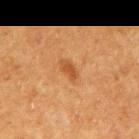This lesion was catalogued during total-body skin photography and was not selected for biopsy.
A 15 mm close-up tile from a total-body photography series done for melanoma screening.
A male patient, in their mid-70s.
Located on the arm.